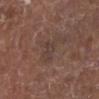biopsy_status: not biopsied; imaged during a skin examination
lighting: white-light
image:
  source: total-body photography crop
  field_of_view_mm: 15
patient:
  sex: male
  age_approx: 75
lesion_size:
  long_diameter_mm_approx: 2.5
automated_metrics:
  area_mm2_approx: 3.0
  eccentricity: 0.75
  shape_asymmetry: 0.5
  nevus_likeness_0_100: 0
  lesion_detection_confidence_0_100: 100
site: left lower leg